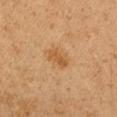notes = catalogued during a skin exam; not biopsied | diameter = about 3 mm | automated lesion analysis = a lesion color around L≈48 a*≈19 b*≈37 in CIELAB | subject = female, aged 18–22 | imaging modality = 15 mm crop, total-body photography | body site = the right upper arm.